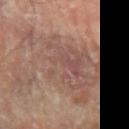Case summary:
– notes — total-body-photography surveillance lesion; no biopsy
– subject — male, in their mid-80s or older
– imaging modality — ~15 mm crop, total-body skin-cancer survey
– location — the left forearm
– image-analysis metrics — a mean CIELAB color near L≈50 a*≈18 b*≈23, about 7 CIELAB-L* units darker than the surrounding skin, and a normalized border contrast of about 5.5; a border-irregularity rating of about 8/10
– diameter — about 10 mm
– illumination — cross-polarized illumination